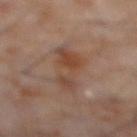The lesion was photographed on a routine skin check and not biopsied; there is no pathology result. A male subject aged approximately 60. The total-body-photography lesion software estimated a mean CIELAB color near L≈41 a*≈17 b*≈26, a lesion–skin lightness drop of about 7, and a normalized border contrast of about 6.5. The software also gave a classifier nevus-likeness of about 15/100 and a lesion-detection confidence of about 100/100. The lesion is on the abdomen. A region of skin cropped from a whole-body photographic capture, roughly 15 mm wide. Measured at roughly 6.5 mm in maximum diameter.